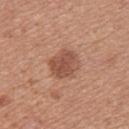Captured during whole-body skin photography for melanoma surveillance; the lesion was not biopsied. A female patient aged 33–37. The recorded lesion diameter is about 3.5 mm. Imaged with white-light lighting. From the back. A roughly 15 mm field-of-view crop from a total-body skin photograph.